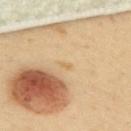Part of a total-body skin-imaging series; this lesion was reviewed on a skin check and was not flagged for biopsy.
Longest diameter approximately 1.5 mm.
This is a cross-polarized tile.
A female patient about 60 years old.
Automated tile analysis of the lesion measured a lesion color around L≈69 a*≈15 b*≈43 in CIELAB, about 6 CIELAB-L* units darker than the surrounding skin, and a normalized border contrast of about 5. It also reported a border-irregularity rating of about 3.5/10 and a within-lesion color-variation index near 0/10.
A close-up tile cropped from a whole-body skin photograph, about 15 mm across.
The lesion is located on the upper back.